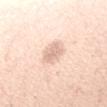Q: Is there a histopathology result?
A: imaged on a skin check; not biopsied
Q: What lighting was used for the tile?
A: white-light
Q: What is the imaging modality?
A: total-body-photography crop, ~15 mm field of view
Q: Lesion location?
A: the left forearm
Q: Patient demographics?
A: female, aged 23 to 27